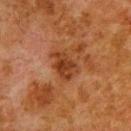biopsy status: no biopsy performed (imaged during a skin exam)
illumination: cross-polarized illumination
subject: male, in their 80s
site: the back
image: total-body-photography crop, ~15 mm field of view